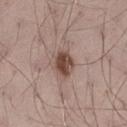Notes:
– notes · no biopsy performed (imaged during a skin exam)
– patient · male, roughly 70 years of age
– automated lesion analysis · an outline eccentricity of about 0.65 (0 = round, 1 = elongated) and a shape-asymmetry score of about 0.1 (0 = symmetric); a lesion color around L≈47 a*≈18 b*≈24 in CIELAB, roughly 14 lightness units darker than nearby skin, and a normalized lesion–skin contrast near 10; a peripheral color-asymmetry measure near 1.5; an automated nevus-likeness rating near 90 out of 100 and a detector confidence of about 100 out of 100 that the crop contains a lesion
– lighting · white-light
– size · ~3 mm (longest diameter)
– site · the left thigh
– image source · ~15 mm tile from a whole-body skin photo